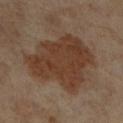The lesion was tiled from a total-body skin photograph and was not biopsied. A 15 mm close-up tile from a total-body photography series done for melanoma screening. A female patient, aged 63 to 67. The lesion is located on the left leg. Measured at roughly 8 mm in maximum diameter.